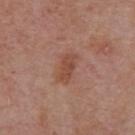| feature | finding |
|---|---|
| biopsy status | total-body-photography surveillance lesion; no biopsy |
| subject | female, aged around 60 |
| image | total-body-photography crop, ~15 mm field of view |
| lighting | white-light |
| anatomic site | the upper back |
| image-analysis metrics | an area of roughly 5.5 mm² and a shape-asymmetry score of about 0.25 (0 = symmetric); border irregularity of about 3 on a 0–10 scale |
| size | about 3.5 mm |